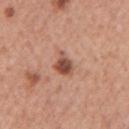{
  "biopsy_status": "not biopsied; imaged during a skin examination",
  "patient": {
    "sex": "female",
    "age_approx": 60
  },
  "site": "left upper arm",
  "image": {
    "source": "total-body photography crop",
    "field_of_view_mm": 15
  },
  "automated_metrics": {
    "eccentricity": 0.5,
    "shape_asymmetry": 0.3,
    "cielab_L": 51,
    "cielab_a": 24,
    "cielab_b": 29,
    "vs_skin_darker_L": 14.0,
    "vs_skin_contrast_norm": 9.5,
    "border_irregularity_0_10": 3.0,
    "color_variation_0_10": 5.0,
    "peripheral_color_asymmetry": 1.5
  },
  "lesion_size": {
    "long_diameter_mm_approx": 3.0
  }
}